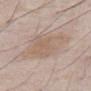Image and clinical context: Cropped from a total-body skin-imaging series; the visible field is about 15 mm. The subject is a male in their mid-50s. This is a white-light tile. Located on the front of the torso. The recorded lesion diameter is about 5.5 mm.